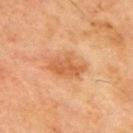Recorded during total-body skin imaging; not selected for excision or biopsy.